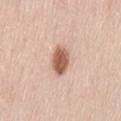image-analysis metrics = a lesion area of about 7.5 mm² and an eccentricity of roughly 0.8; a border-irregularity rating of about 2/10 and a color-variation rating of about 4/10
subject = female, about 40 years old
image source = ~15 mm tile from a whole-body skin photo
lesion diameter = ≈4 mm
body site = the lower back
lighting = white-light